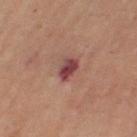Imaged during a routine full-body skin examination; the lesion was not biopsied and no histopathology is available.
Automated image analysis of the tile measured a footprint of about 5 mm², a shape eccentricity near 0.8, and two-axis asymmetry of about 0.25. The analysis additionally found a border-irregularity rating of about 2/10, internal color variation of about 5.5 on a 0–10 scale, and peripheral color asymmetry of about 2. It also reported an automated nevus-likeness rating near 0 out of 100.
A lesion tile, about 15 mm wide, cut from a 3D total-body photograph.
A female subject aged 68 to 72.
Longest diameter approximately 3 mm.
From the left thigh.